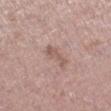Recorded during total-body skin imaging; not selected for excision or biopsy.
Captured under white-light illumination.
Automated image analysis of the tile measured a border-irregularity index near 6.5/10, a color-variation rating of about 1/10, and peripheral color asymmetry of about 0. The software also gave a classifier nevus-likeness of about 0/100 and lesion-presence confidence of about 100/100.
The recorded lesion diameter is about 3.5 mm.
A 15 mm crop from a total-body photograph taken for skin-cancer surveillance.
The patient is a female aged approximately 40.
On the right lower leg.